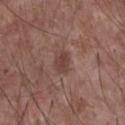Q: Is there a histopathology result?
A: catalogued during a skin exam; not biopsied
Q: Lesion location?
A: the chest
Q: What kind of image is this?
A: 15 mm crop, total-body photography
Q: Patient demographics?
A: male, aged approximately 60